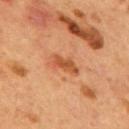Context: The lesion is on the mid back. Longest diameter approximately 3.5 mm. The tile uses cross-polarized illumination. A region of skin cropped from a whole-body photographic capture, roughly 15 mm wide. The subject is a male aged 53–57.